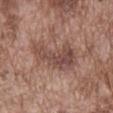Case summary:
- biopsy status · total-body-photography surveillance lesion; no biopsy
- lighting · white-light illumination
- lesion size · about 5.5 mm
- location · the abdomen
- imaging modality · ~15 mm crop, total-body skin-cancer survey
- patient · male, in their mid- to late 70s
- image-analysis metrics · a border-irregularity rating of about 5.5/10